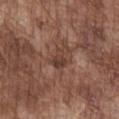workup: imaged on a skin check; not biopsied
lighting: white-light
site: the chest
image-analysis metrics: a lesion area of about 4 mm², an outline eccentricity of about 0.65 (0 = round, 1 = elongated), and a shape-asymmetry score of about 0.4 (0 = symmetric); internal color variation of about 2.5 on a 0–10 scale and radial color variation of about 0.5
acquisition: ~15 mm crop, total-body skin-cancer survey
patient: male, aged 73–77
lesion size: about 2.5 mm